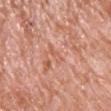Q: How was the tile lit?
A: white-light illumination
Q: Where on the body is the lesion?
A: the chest
Q: How large is the lesion?
A: about 3 mm
Q: What is the imaging modality?
A: 15 mm crop, total-body photography
Q: Patient demographics?
A: male, aged 78–82
Q: Automated lesion metrics?
A: an eccentricity of roughly 0.95 and two-axis asymmetry of about 0.5; a mean CIELAB color near L≈60 a*≈26 b*≈33, about 6 CIELAB-L* units darker than the surrounding skin, and a normalized border contrast of about 4.5; a lesion-detection confidence of about 75/100